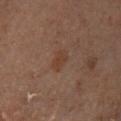No biopsy was performed on this lesion — it was imaged during a full skin examination and was not determined to be concerning. On the right lower leg. Imaged with cross-polarized lighting. A region of skin cropped from a whole-body photographic capture, roughly 15 mm wide. The patient is a female in their 50s.